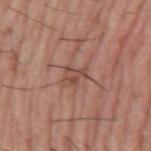Automated image analysis of the tile measured an outline eccentricity of about 0.75 (0 = round, 1 = elongated). It also reported a border-irregularity rating of about 5/10.
Longest diameter approximately 2.5 mm.
A 15 mm close-up extracted from a 3D total-body photography capture.
The lesion is located on the arm.
The tile uses white-light illumination.
A male subject aged 53–57.